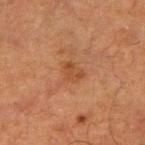| feature | finding |
|---|---|
| patient | male, in their mid- to late 60s |
| lesion size | ~2.5 mm (longest diameter) |
| illumination | cross-polarized illumination |
| acquisition | total-body-photography crop, ~15 mm field of view |
| TBP lesion metrics | border irregularity of about 2 on a 0–10 scale, a within-lesion color-variation index near 2/10, and a peripheral color-asymmetry measure near 1 |
| body site | the arm |